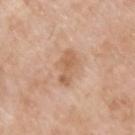follow-up: total-body-photography surveillance lesion; no biopsy
diameter: ≈3.5 mm
acquisition: 15 mm crop, total-body photography
anatomic site: the upper back
illumination: white-light
patient: male, aged around 65